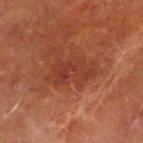Impression:
Imaged during a routine full-body skin examination; the lesion was not biopsied and no histopathology is available.
Clinical summary:
The subject is a male aged 68 to 72. An algorithmic analysis of the crop reported an average lesion color of about L≈31 a*≈26 b*≈27 (CIELAB), roughly 6 lightness units darker than nearby skin, and a normalized lesion–skin contrast near 6. The analysis additionally found border irregularity of about 7.5 on a 0–10 scale, a within-lesion color-variation index near 2.5/10, and peripheral color asymmetry of about 0.5. And it measured a nevus-likeness score of about 5/100 and a lesion-detection confidence of about 100/100. A roughly 15 mm field-of-view crop from a total-body skin photograph. The lesion is on the right lower leg. About 5.5 mm across. The tile uses cross-polarized illumination.